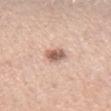Clinical impression:
Part of a total-body skin-imaging series; this lesion was reviewed on a skin check and was not flagged for biopsy.
Background:
A female patient roughly 45 years of age. Imaged with white-light lighting. From the left forearm. A lesion tile, about 15 mm wide, cut from a 3D total-body photograph. Approximately 2.5 mm at its widest.